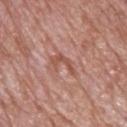  biopsy_status: not biopsied; imaged during a skin examination
  patient:
    sex: male
    age_approx: 70
  automated_metrics:
    area_mm2_approx: 4.0
    eccentricity: 0.85
    shape_asymmetry: 0.65
    cielab_L: 52
    cielab_a: 25
    cielab_b: 28
    vs_skin_darker_L: 8.0
    vs_skin_contrast_norm: 6.5
    border_irregularity_0_10: 7.5
    color_variation_0_10: 0.0
    peripheral_color_asymmetry: 0.0
  image:
    source: total-body photography crop
    field_of_view_mm: 15
  site: back
  lighting: white-light